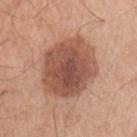Assessment: The lesion was tiled from a total-body skin photograph and was not biopsied. Background: The total-body-photography lesion software estimated an eccentricity of roughly 0.4. And it measured a normalized lesion–skin contrast near 9.5. Longest diameter approximately 7 mm. A 15 mm crop from a total-body photograph taken for skin-cancer surveillance. The patient is a male aged 68 to 72. The lesion is on the arm.